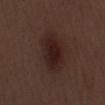Recorded during total-body skin imaging; not selected for excision or biopsy. This is a white-light tile. A male patient, roughly 70 years of age. Measured at roughly 5 mm in maximum diameter. Located on the left forearm. A region of skin cropped from a whole-body photographic capture, roughly 15 mm wide.